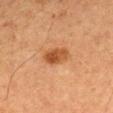<tbp_lesion>
<biopsy_status>not biopsied; imaged during a skin examination</biopsy_status>
<lighting>cross-polarized</lighting>
<patient>
  <sex>male</sex>
  <age_approx>65</age_approx>
</patient>
<site>mid back</site>
<image>
  <source>total-body photography crop</source>
  <field_of_view_mm>15</field_of_view_mm>
</image>
<lesion_size>
  <long_diameter_mm_approx>4.0</long_diameter_mm_approx>
</lesion_size>
</tbp_lesion>